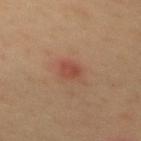The lesion was tiled from a total-body skin photograph and was not biopsied. The tile uses cross-polarized illumination. From the mid back. Cropped from a whole-body photographic skin survey; the tile spans about 15 mm. The subject is a female aged 48–52. An algorithmic analysis of the crop reported an area of roughly 3.5 mm², a shape eccentricity near 0.65, and two-axis asymmetry of about 0.25. The analysis additionally found a lesion color around L≈46 a*≈26 b*≈29 in CIELAB, roughly 8 lightness units darker than nearby skin, and a normalized lesion–skin contrast near 6.5.